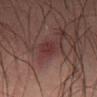Impression: No biopsy was performed on this lesion — it was imaged during a full skin examination and was not determined to be concerning. Acquisition and patient details: A male subject, aged 48–52. This is a cross-polarized tile. A 15 mm close-up tile from a total-body photography series done for melanoma screening. From the left thigh.